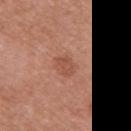{
  "biopsy_status": "not biopsied; imaged during a skin examination",
  "image": {
    "source": "total-body photography crop",
    "field_of_view_mm": 15
  },
  "site": "left upper arm",
  "automated_metrics": {
    "area_mm2_approx": 4.5,
    "eccentricity": 0.7,
    "shape_asymmetry": 0.25,
    "vs_skin_darker_L": 7.0,
    "vs_skin_contrast_norm": 5.5,
    "nevus_likeness_0_100": 10,
    "lesion_detection_confidence_0_100": 100
  },
  "patient": {
    "sex": "female",
    "age_approx": 65
  },
  "lighting": "white-light"
}